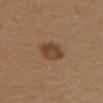Recorded during total-body skin imaging; not selected for excision or biopsy. From the upper back. Imaged with white-light lighting. A female patient aged 38 to 42. Measured at roughly 3.5 mm in maximum diameter. Cropped from a whole-body photographic skin survey; the tile spans about 15 mm.